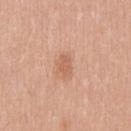<lesion>
<biopsy_status>not biopsied; imaged during a skin examination</biopsy_status>
<patient>
  <sex>male</sex>
  <age_approx>45</age_approx>
</patient>
<automated_metrics>
  <area_mm2_approx>3.5</area_mm2_approx>
  <eccentricity>0.8</eccentricity>
  <shape_asymmetry>0.3</shape_asymmetry>
  <cielab_L>61</cielab_L>
  <cielab_a>24</cielab_a>
  <cielab_b>33</cielab_b>
  <vs_skin_darker_L>8.0</vs_skin_darker_L>
  <nevus_likeness_0_100>5</nevus_likeness_0_100>
  <lesion_detection_confidence_0_100>100</lesion_detection_confidence_0_100>
</automated_metrics>
<image>
  <source>total-body photography crop</source>
  <field_of_view_mm>15</field_of_view_mm>
</image>
<lighting>white-light</lighting>
<site>mid back</site>
<lesion_size>
  <long_diameter_mm_approx>2.5</long_diameter_mm_approx>
</lesion_size>
</lesion>